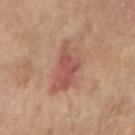<record>
  <biopsy_status>not biopsied; imaged during a skin examination</biopsy_status>
  <lighting>cross-polarized</lighting>
  <patient>
    <sex>female</sex>
    <age_approx>65</age_approx>
  </patient>
  <lesion_size>
    <long_diameter_mm_approx>6.0</long_diameter_mm_approx>
  </lesion_size>
  <automated_metrics>
    <eccentricity>0.8</eccentricity>
    <shape_asymmetry>0.3</shape_asymmetry>
    <vs_skin_darker_L>9.0</vs_skin_darker_L>
    <vs_skin_contrast_norm>7.0</vs_skin_contrast_norm>
    <nevus_likeness_0_100>25</nevus_likeness_0_100>
    <lesion_detection_confidence_0_100>100</lesion_detection_confidence_0_100>
  </automated_metrics>
  <image>
    <source>total-body photography crop</source>
    <field_of_view_mm>15</field_of_view_mm>
  </image>
  <site>arm</site>
</record>